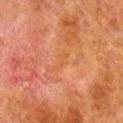Clinical summary:
About 2.5 mm across. The lesion is on the right lower leg. Imaged with cross-polarized lighting. A male subject about 80 years old. A 15 mm crop from a total-body photograph taken for skin-cancer surveillance.